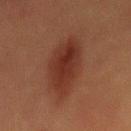{
  "biopsy_status": "not biopsied; imaged during a skin examination",
  "image": {
    "source": "total-body photography crop",
    "field_of_view_mm": 15
  },
  "lighting": "cross-polarized",
  "patient": {
    "sex": "male",
    "age_approx": 40
  },
  "lesion_size": {
    "long_diameter_mm_approx": 8.0
  },
  "site": "mid back"
}